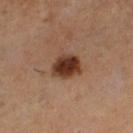  biopsy_status: not biopsied; imaged during a skin examination
  lighting: cross-polarized
  site: leg
  patient:
    sex: female
    age_approx: 55
  image:
    source: total-body photography crop
    field_of_view_mm: 15